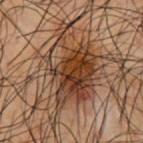* follow-up · imaged on a skin check; not biopsied
* location · the front of the torso
* patient · male, roughly 50 years of age
* automated lesion analysis · a lesion area of about 29 mm², an outline eccentricity of about 0.7 (0 = round, 1 = elongated), and a shape-asymmetry score of about 0.3 (0 = symmetric); a mean CIELAB color near L≈29 a*≈15 b*≈24, about 10 CIELAB-L* units darker than the surrounding skin, and a lesion-to-skin contrast of about 10.5 (normalized; higher = more distinct); a border-irregularity rating of about 4.5/10, internal color variation of about 8.5 on a 0–10 scale, and peripheral color asymmetry of about 3; a classifier nevus-likeness of about 65/100 and a detector confidence of about 100 out of 100 that the crop contains a lesion
* lighting · cross-polarized illumination
* image · 15 mm crop, total-body photography
* lesion size · ~8.5 mm (longest diameter)